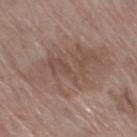Case summary:
* workup: total-body-photography surveillance lesion; no biopsy
* lesion diameter: about 10 mm
* illumination: white-light illumination
* acquisition: total-body-photography crop, ~15 mm field of view
* TBP lesion metrics: a lesion area of about 44 mm² and a symmetry-axis asymmetry near 0.2; a border-irregularity index near 5/10, a color-variation rating of about 4/10, and peripheral color asymmetry of about 1.5; an automated nevus-likeness rating near 0 out of 100 and a lesion-detection confidence of about 100/100
* location: the right thigh
* patient: female, roughly 70 years of age